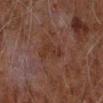Captured during whole-body skin photography for melanoma surveillance; the lesion was not biopsied. A male subject, in their 60s. The lesion is located on the left forearm. A 15 mm crop from a total-body photograph taken for skin-cancer surveillance.